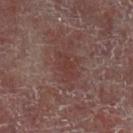Q: Is there a histopathology result?
A: total-body-photography surveillance lesion; no biopsy
Q: Patient demographics?
A: male, aged approximately 60
Q: What is the anatomic site?
A: the left lower leg
Q: What lighting was used for the tile?
A: cross-polarized
Q: Automated lesion metrics?
A: a footprint of about 4.5 mm², a shape eccentricity near 0.85, and two-axis asymmetry of about 0.25; a mean CIELAB color near L≈32 a*≈19 b*≈19, about 5 CIELAB-L* units darker than the surrounding skin, and a normalized lesion–skin contrast near 5
Q: What kind of image is this?
A: total-body-photography crop, ~15 mm field of view
Q: What is the lesion's diameter?
A: about 3.5 mm